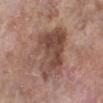Recorded during total-body skin imaging; not selected for excision or biopsy. The tile uses white-light illumination. A female patient, aged approximately 75. Located on the leg. The lesion's longest dimension is about 7 mm. A roughly 15 mm field-of-view crop from a total-body skin photograph.